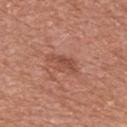notes: total-body-photography surveillance lesion; no biopsy | subject: female, aged around 65 | image: ~15 mm tile from a whole-body skin photo | anatomic site: the left upper arm | lighting: white-light.